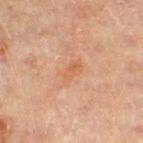Clinical impression: Part of a total-body skin-imaging series; this lesion was reviewed on a skin check and was not flagged for biopsy. Background: A male patient aged 83 or over. The lesion is located on the right thigh. A lesion tile, about 15 mm wide, cut from a 3D total-body photograph.